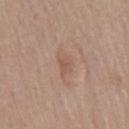Q: Was a biopsy performed?
A: imaged on a skin check; not biopsied
Q: Automated lesion metrics?
A: a border-irregularity rating of about 3.5/10 and a within-lesion color-variation index near 1.5/10
Q: Where on the body is the lesion?
A: the chest
Q: What kind of image is this?
A: ~15 mm crop, total-body skin-cancer survey
Q: What are the patient's age and sex?
A: male, in their mid- to late 70s